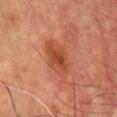– workup — no biopsy performed (imaged during a skin exam)
– imaging modality — ~15 mm crop, total-body skin-cancer survey
– body site — the chest
– lighting — cross-polarized illumination
– subject — male, about 55 years old